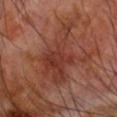Clinical impression: The lesion was tiled from a total-body skin photograph and was not biopsied. Image and clinical context: A male subject approximately 70 years of age. A roughly 15 mm field-of-view crop from a total-body skin photograph. Imaged with cross-polarized lighting. The lesion is located on the right forearm. Approximately 8 mm at its widest.